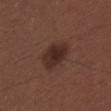{"biopsy_status": "not biopsied; imaged during a skin examination", "patient": {"sex": "male", "age_approx": 30}, "lesion_size": {"long_diameter_mm_approx": 4.5}, "image": {"source": "total-body photography crop", "field_of_view_mm": 15}, "site": "upper back"}